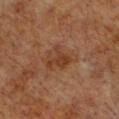Clinical impression: The lesion was photographed on a routine skin check and not biopsied; there is no pathology result. Context: Imaged with cross-polarized lighting. On the front of the torso. A region of skin cropped from a whole-body photographic capture, roughly 15 mm wide. About 3 mm across. A female patient, in their mid-50s.